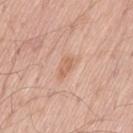Q: Was this lesion biopsied?
A: total-body-photography surveillance lesion; no biopsy
Q: What is the anatomic site?
A: the left thigh
Q: What is the imaging modality?
A: ~15 mm tile from a whole-body skin photo
Q: What is the lesion's diameter?
A: ~3 mm (longest diameter)
Q: Who is the patient?
A: male, aged around 60